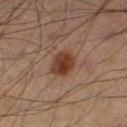Notes:
* follow-up — no biopsy performed (imaged during a skin exam)
* lesion size — ≈3.5 mm
* imaging modality — ~15 mm crop, total-body skin-cancer survey
* location — the leg
* subject — male, in their 70s
* automated metrics — an area of roughly 7 mm², a shape eccentricity near 0.7, and a symmetry-axis asymmetry near 0.15; a classifier nevus-likeness of about 95/100 and lesion-presence confidence of about 100/100
* illumination — cross-polarized illumination